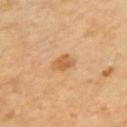<tbp_lesion>
<image>
  <source>total-body photography crop</source>
  <field_of_view_mm>15</field_of_view_mm>
</image>
<patient>
  <age_approx>65</age_approx>
</patient>
<site>arm</site>
<lesion_size>
  <long_diameter_mm_approx>3.0</long_diameter_mm_approx>
</lesion_size>
<lighting>cross-polarized</lighting>
</tbp_lesion>